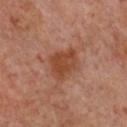Imaged during a routine full-body skin examination; the lesion was not biopsied and no histopathology is available. From the chest. This image is a 15 mm lesion crop taken from a total-body photograph. The subject is a female in their 60s.